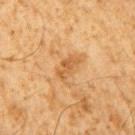Case summary:
* notes · catalogued during a skin exam; not biopsied
* lesion diameter · about 3.5 mm
* image · ~15 mm tile from a whole-body skin photo
* subject · male, approximately 60 years of age
* tile lighting · cross-polarized
* automated metrics · a lesion area of about 5 mm² and two-axis asymmetry of about 0.3; an average lesion color of about L≈49 a*≈19 b*≈37 (CIELAB), a lesion–skin lightness drop of about 8, and a normalized border contrast of about 6; a nevus-likeness score of about 0/100 and lesion-presence confidence of about 100/100
* location · the right upper arm